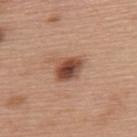No biopsy was performed on this lesion — it was imaged during a full skin examination and was not determined to be concerning. The lesion's longest dimension is about 3.5 mm. A region of skin cropped from a whole-body photographic capture, roughly 15 mm wide. On the back. The subject is a female approximately 60 years of age.